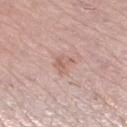The lesion was tiled from a total-body skin photograph and was not biopsied.